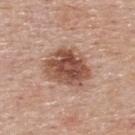{"lesion_size": {"long_diameter_mm_approx": 5.5}, "site": "upper back", "image": {"source": "total-body photography crop", "field_of_view_mm": 15}, "patient": {"sex": "female", "age_approx": 65}, "lighting": "white-light", "automated_metrics": {"cielab_L": 50, "cielab_a": 22, "cielab_b": 28, "vs_skin_darker_L": 15.0, "vs_skin_contrast_norm": 10.0, "nevus_likeness_0_100": 60, "lesion_detection_confidence_0_100": 100}}